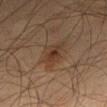follow-up: no biopsy performed (imaged during a skin exam).